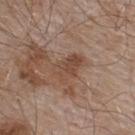<tbp_lesion>
  <biopsy_status>not biopsied; imaged during a skin examination</biopsy_status>
  <lesion_size>
    <long_diameter_mm_approx>4.0</long_diameter_mm_approx>
  </lesion_size>
  <site>upper back</site>
  <patient>
    <sex>male</sex>
    <age_approx>55</age_approx>
  </patient>
  <image>
    <source>total-body photography crop</source>
    <field_of_view_mm>15</field_of_view_mm>
  </image>
</tbp_lesion>